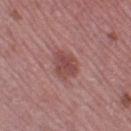workup = imaged on a skin check; not biopsied
TBP lesion metrics = a footprint of about 7 mm² and an eccentricity of roughly 0.65; a lesion-detection confidence of about 100/100
lesion size = ≈4 mm
imaging modality = 15 mm crop, total-body photography
body site = the left thigh
patient = female, in their mid-40s
tile lighting = white-light